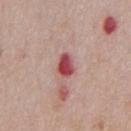biopsy status: imaged on a skin check; not biopsied | anatomic site: the front of the torso | automated metrics: a lesion area of about 5 mm², a shape eccentricity near 0.6, and a symmetry-axis asymmetry near 0.2; about 16 CIELAB-L* units darker than the surrounding skin and a lesion-to-skin contrast of about 11 (normalized; higher = more distinct); a classifier nevus-likeness of about 0/100 and a detector confidence of about 100 out of 100 that the crop contains a lesion | lighting: white-light | subject: male, roughly 60 years of age | image: ~15 mm tile from a whole-body skin photo | diameter: ~2.5 mm (longest diameter).